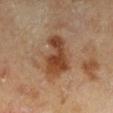notes: imaged on a skin check; not biopsied
illumination: cross-polarized illumination
subject: male, aged 63–67
automated lesion analysis: a shape-asymmetry score of about 0.3 (0 = symmetric); a detector confidence of about 100 out of 100 that the crop contains a lesion
body site: the left lower leg
image: ~15 mm tile from a whole-body skin photo